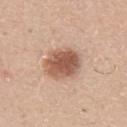Findings:
• biopsy status · catalogued during a skin exam; not biopsied
• imaging modality · total-body-photography crop, ~15 mm field of view
• lesion diameter · ≈4.5 mm
• site · the right upper arm
• subject · male, in their 60s
• illumination · white-light